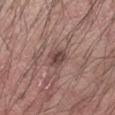Imaged during a routine full-body skin examination; the lesion was not biopsied and no histopathology is available.
A male patient, aged 23–27.
A region of skin cropped from a whole-body photographic capture, roughly 15 mm wide.
The lesion is on the arm.